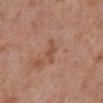{
  "biopsy_status": "not biopsied; imaged during a skin examination",
  "image": {
    "source": "total-body photography crop",
    "field_of_view_mm": 15
  },
  "site": "abdomen",
  "patient": {
    "sex": "male",
    "age_approx": 70
  },
  "lesion_size": {
    "long_diameter_mm_approx": 3.0
  }
}